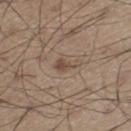This lesion was catalogued during total-body skin photography and was not selected for biopsy. A male subject, aged 53 to 57. A close-up tile cropped from a whole-body skin photograph, about 15 mm across. Located on the leg.